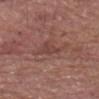The lesion was tiled from a total-body skin photograph and was not biopsied.
Captured under white-light illumination.
Longest diameter approximately 2.5 mm.
The patient is a male aged 78 to 82.
This image is a 15 mm lesion crop taken from a total-body photograph.
From the head or neck.